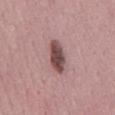{"biopsy_status": "not biopsied; imaged during a skin examination", "lesion_size": {"long_diameter_mm_approx": 5.0}, "automated_metrics": {"border_irregularity_0_10": 3.0, "color_variation_0_10": 4.0, "peripheral_color_asymmetry": 1.0, "nevus_likeness_0_100": 100, "lesion_detection_confidence_0_100": 100}, "patient": {"sex": "male", "age_approx": 50}, "site": "mid back", "lighting": "white-light", "image": {"source": "total-body photography crop", "field_of_view_mm": 15}}